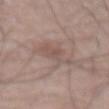notes: catalogued during a skin exam; not biopsied | size: ≈5.5 mm | patient: male, aged 68 to 72 | illumination: white-light illumination | image: total-body-photography crop, ~15 mm field of view | location: the abdomen.